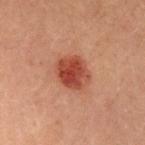follow-up: no biopsy performed (imaged during a skin exam) | location: the upper back | TBP lesion metrics: a lesion area of about 11 mm²; a lesion color around L≈37 a*≈25 b*≈27 in CIELAB, a lesion–skin lightness drop of about 11, and a lesion-to-skin contrast of about 9 (normalized; higher = more distinct) | lesion size: ~4 mm (longest diameter) | imaging modality: total-body-photography crop, ~15 mm field of view | illumination: cross-polarized | subject: female, aged approximately 30.